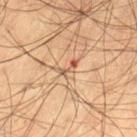follow-up = imaged on a skin check; not biopsied | lighting = cross-polarized | patient = male, aged around 65 | body site = the left thigh | image source = total-body-photography crop, ~15 mm field of view | automated lesion analysis = a footprint of about 2.5 mm² and an eccentricity of roughly 0.9; internal color variation of about 0 on a 0–10 scale and peripheral color asymmetry of about 0.